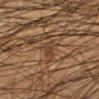subject: male, aged 43 to 47 | location: the leg | imaging modality: ~15 mm crop, total-body skin-cancer survey.